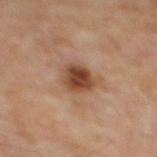Assessment:
Imaged during a routine full-body skin examination; the lesion was not biopsied and no histopathology is available.
Acquisition and patient details:
The lesion-visualizer software estimated a lesion area of about 9 mm², a shape eccentricity near 0.6, and a symmetry-axis asymmetry near 0.25. The software also gave roughly 13 lightness units darker than nearby skin and a lesion-to-skin contrast of about 10 (normalized; higher = more distinct). A 15 mm close-up tile from a total-body photography series done for melanoma screening. The recorded lesion diameter is about 4 mm. On the mid back. The subject is a male in their mid- to late 60s.